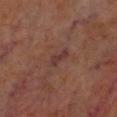<lesion>
<biopsy_status>not biopsied; imaged during a skin examination</biopsy_status>
<site>left lower leg</site>
<automated_metrics>
  <cielab_L>28</cielab_L>
  <cielab_a>16</cielab_a>
  <cielab_b>17</cielab_b>
  <vs_skin_darker_L>6.0</vs_skin_darker_L>
  <vs_skin_contrast_norm>7.5</vs_skin_contrast_norm>
  <nevus_likeness_0_100>0</nevus_likeness_0_100>
  <lesion_detection_confidence_0_100>95</lesion_detection_confidence_0_100>
</automated_metrics>
<image>
  <source>total-body photography crop</source>
  <field_of_view_mm>15</field_of_view_mm>
</image>
<lesion_size>
  <long_diameter_mm_approx>3.0</long_diameter_mm_approx>
</lesion_size>
<patient>
  <sex>male</sex>
  <age_approx>70</age_approx>
</patient>
<lighting>cross-polarized</lighting>
</lesion>